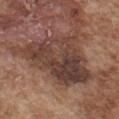Image and clinical context: From the chest. Longest diameter approximately 9.5 mm. A male subject, in their mid- to late 70s. Imaged with white-light lighting. A roughly 15 mm field-of-view crop from a total-body skin photograph.